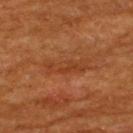{
  "patient": {
    "sex": "male",
    "age_approx": 60
  },
  "site": "upper back",
  "lighting": "cross-polarized",
  "image": {
    "source": "total-body photography crop",
    "field_of_view_mm": 15
  },
  "lesion_size": {
    "long_diameter_mm_approx": 5.0
  }
}